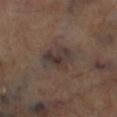biopsy_status: not biopsied; imaged during a skin examination
site: right lower leg
image:
  source: total-body photography crop
  field_of_view_mm: 15
lesion_size:
  long_diameter_mm_approx: 4.5
lighting: cross-polarized